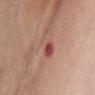The lesion was photographed on a routine skin check and not biopsied; there is no pathology result. About 3 mm across. Automated image analysis of the tile measured an area of roughly 5.5 mm². The analysis additionally found a mean CIELAB color near L≈51 a*≈26 b*≈25. And it measured border irregularity of about 2.5 on a 0–10 scale and internal color variation of about 10 on a 0–10 scale. The analysis additionally found a classifier nevus-likeness of about 0/100 and a detector confidence of about 100 out of 100 that the crop contains a lesion. The subject is a female aged around 70. The lesion is located on the chest. A close-up tile cropped from a whole-body skin photograph, about 15 mm across.